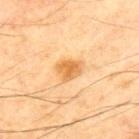  site: chest
  patient:
    sex: male
    age_approx: 70
  lighting: cross-polarized
  lesion_size:
    long_diameter_mm_approx: 3.5
  image:
    source: total-body photography crop
    field_of_view_mm: 15